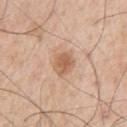No biopsy was performed on this lesion — it was imaged during a full skin examination and was not determined to be concerning.